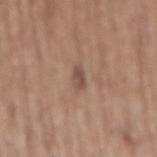Impression: Captured during whole-body skin photography for melanoma surveillance; the lesion was not biopsied. Image and clinical context: The tile uses white-light illumination. This image is a 15 mm lesion crop taken from a total-body photograph. On the mid back. The subject is a female in their mid-60s.